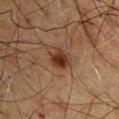Captured during whole-body skin photography for melanoma surveillance; the lesion was not biopsied.
Automated image analysis of the tile measured an area of roughly 4.5 mm² and two-axis asymmetry of about 0.35. It also reported a mean CIELAB color near L≈26 a*≈18 b*≈24, about 10 CIELAB-L* units darker than the surrounding skin, and a normalized border contrast of about 11. The analysis additionally found border irregularity of about 3 on a 0–10 scale, a within-lesion color-variation index near 3/10, and radial color variation of about 1.
The lesion is on the chest.
A roughly 15 mm field-of-view crop from a total-body skin photograph.
The recorded lesion diameter is about 2.5 mm.
A male subject roughly 60 years of age.
Imaged with cross-polarized lighting.